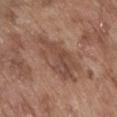Assessment: No biopsy was performed on this lesion — it was imaged during a full skin examination and was not determined to be concerning. Acquisition and patient details: Cropped from a whole-body photographic skin survey; the tile spans about 15 mm. A male patient, about 75 years old. Captured under white-light illumination. From the abdomen. Measured at roughly 7 mm in maximum diameter. The total-body-photography lesion software estimated an automated nevus-likeness rating near 0 out of 100 and lesion-presence confidence of about 90/100.